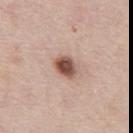Impression:
Imaged during a routine full-body skin examination; the lesion was not biopsied and no histopathology is available.
Image and clinical context:
Captured under white-light illumination. Measured at roughly 3 mm in maximum diameter. A male patient about 40 years old. The lesion is located on the mid back. A lesion tile, about 15 mm wide, cut from a 3D total-body photograph.